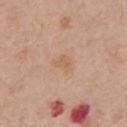Recorded during total-body skin imaging; not selected for excision or biopsy.
The total-body-photography lesion software estimated an average lesion color of about L≈61 a*≈18 b*≈32 (CIELAB), about 6 CIELAB-L* units darker than the surrounding skin, and a normalized lesion–skin contrast near 5.
Captured under white-light illumination.
The lesion is on the chest.
Longest diameter approximately 3 mm.
A roughly 15 mm field-of-view crop from a total-body skin photograph.
The subject is a male in their 60s.